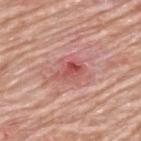Impression:
Recorded during total-body skin imaging; not selected for excision or biopsy.
Background:
The lesion is on the upper back. The lesion-visualizer software estimated a shape eccentricity near 0.85 and a symmetry-axis asymmetry near 0.3. The analysis additionally found about 10 CIELAB-L* units darker than the surrounding skin and a lesion-to-skin contrast of about 7 (normalized; higher = more distinct). And it measured a border-irregularity index near 3/10, a color-variation rating of about 6.5/10, and a peripheral color-asymmetry measure near 2.5. And it measured a classifier nevus-likeness of about 0/100 and lesion-presence confidence of about 100/100. A region of skin cropped from a whole-body photographic capture, roughly 15 mm wide. A male subject approximately 80 years of age. This is a white-light tile.